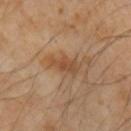This lesion was catalogued during total-body skin photography and was not selected for biopsy. A 15 mm crop from a total-body photograph taken for skin-cancer surveillance. From the arm. A female subject aged 43–47.